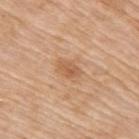This lesion was catalogued during total-body skin photography and was not selected for biopsy. Captured under white-light illumination. Cropped from a whole-body photographic skin survey; the tile spans about 15 mm. Measured at roughly 2.5 mm in maximum diameter. From the right upper arm. An algorithmic analysis of the crop reported a shape-asymmetry score of about 0.25 (0 = symmetric). The analysis additionally found a lesion color around L≈58 a*≈21 b*≈36 in CIELAB, about 9 CIELAB-L* units darker than the surrounding skin, and a normalized lesion–skin contrast near 6. And it measured a border-irregularity rating of about 2/10, internal color variation of about 2.5 on a 0–10 scale, and peripheral color asymmetry of about 1. And it measured a nevus-likeness score of about 50/100 and a detector confidence of about 100 out of 100 that the crop contains a lesion. A female patient aged 73 to 77.